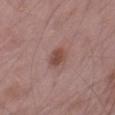Imaged with white-light lighting.
Located on the right thigh.
A male subject, aged 48–52.
The total-body-photography lesion software estimated a shape eccentricity near 0.65 and a shape-asymmetry score of about 0.2 (0 = symmetric). The analysis additionally found an average lesion color of about L≈47 a*≈21 b*≈23 (CIELAB), roughly 10 lightness units darker than nearby skin, and a normalized lesion–skin contrast near 7.5. The analysis additionally found a border-irregularity index near 1.5/10 and a color-variation rating of about 2.5/10. And it measured a classifier nevus-likeness of about 85/100 and lesion-presence confidence of about 100/100.
A lesion tile, about 15 mm wide, cut from a 3D total-body photograph.
Measured at roughly 2.5 mm in maximum diameter.